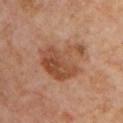Case summary:
– workup — total-body-photography surveillance lesion; no biopsy
– imaging modality — ~15 mm tile from a whole-body skin photo
– anatomic site — the front of the torso
– patient — male, approximately 55 years of age
– automated metrics — a border-irregularity index near 6/10, a color-variation rating of about 5.5/10, and peripheral color asymmetry of about 2; a lesion-detection confidence of about 100/100
– diameter — ≈5.5 mm
– illumination — cross-polarized illumination